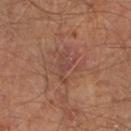tile lighting — cross-polarized
patient — male, in their mid-60s
imaging modality — ~15 mm tile from a whole-body skin photo
size — ≈3.5 mm
site — the right lower leg